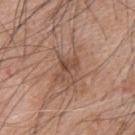notes: no biopsy performed (imaged during a skin exam); diameter: ~3 mm (longest diameter); illumination: white-light illumination; patient: male, about 50 years old; acquisition: total-body-photography crop, ~15 mm field of view; anatomic site: the back.